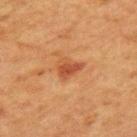Imaged during a routine full-body skin examination; the lesion was not biopsied and no histopathology is available. Imaged with cross-polarized lighting. The lesion is located on the mid back. A female subject, approximately 40 years of age. A region of skin cropped from a whole-body photographic capture, roughly 15 mm wide.